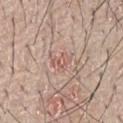No biopsy was performed on this lesion — it was imaged during a full skin examination and was not determined to be concerning. A male subject approximately 65 years of age. A 15 mm close-up extracted from a 3D total-body photography capture. The lesion is located on the chest.